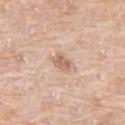<case>
<patient>
  <sex>female</sex>
  <age_approx>75</age_approx>
</patient>
<lighting>white-light</lighting>
<lesion_size>
  <long_diameter_mm_approx>2.5</long_diameter_mm_approx>
</lesion_size>
<image>
  <source>total-body photography crop</source>
  <field_of_view_mm>15</field_of_view_mm>
</image>
<site>leg</site>
<automated_metrics>
  <area_mm2_approx>4.0</area_mm2_approx>
  <eccentricity>0.75</eccentricity>
  <cielab_L>63</cielab_L>
  <cielab_a>19</cielab_a>
  <cielab_b>30</cielab_b>
  <vs_skin_darker_L>10.0</vs_skin_darker_L>
</automated_metrics>
</case>